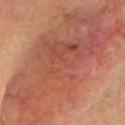Captured during whole-body skin photography for melanoma surveillance; the lesion was not biopsied. This is a cross-polarized tile. Located on the head or neck. Cropped from a whole-body photographic skin survey; the tile spans about 15 mm. The lesion's longest dimension is about 22 mm. A male subject, aged 83 or over.